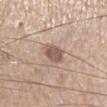Impression: The lesion was tiled from a total-body skin photograph and was not biopsied. Acquisition and patient details: An algorithmic analysis of the crop reported a footprint of about 6.5 mm², a shape eccentricity near 0.75, and two-axis asymmetry of about 0.2. And it measured border irregularity of about 2 on a 0–10 scale, a within-lesion color-variation index near 2.5/10, and a peripheral color-asymmetry measure near 1. A male subject, aged 63–67. A roughly 15 mm field-of-view crop from a total-body skin photograph. The lesion is located on the right lower leg. The tile uses white-light illumination.